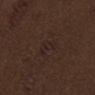Case summary:
- biopsy status — catalogued during a skin exam; not biopsied
- subject — male, approximately 70 years of age
- TBP lesion metrics — a mean CIELAB color near L≈22 a*≈14 b*≈17 and roughly 3 lightness units darker than nearby skin; a border-irregularity rating of about 4/10, internal color variation of about 2.5 on a 0–10 scale, and peripheral color asymmetry of about 1; an automated nevus-likeness rating near 0 out of 100 and lesion-presence confidence of about 100/100
- anatomic site — the front of the torso
- tile lighting — white-light
- acquisition — total-body-photography crop, ~15 mm field of view
- lesion size — ≈3 mm